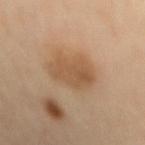{
  "biopsy_status": "not biopsied; imaged during a skin examination",
  "lighting": "cross-polarized",
  "image": {
    "source": "total-body photography crop",
    "field_of_view_mm": 15
  },
  "site": "mid back",
  "patient": {
    "sex": "male",
    "age_approx": 55
  },
  "lesion_size": {
    "long_diameter_mm_approx": 4.5
  }
}